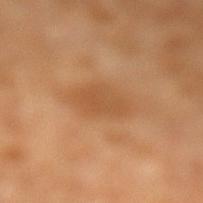Notes:
- follow-up: imaged on a skin check; not biopsied
- lesion diameter: ~4.5 mm (longest diameter)
- TBP lesion metrics: an area of roughly 10 mm², an outline eccentricity of about 0.75 (0 = round, 1 = elongated), and a symmetry-axis asymmetry near 0.15; a mean CIELAB color near L≈52 a*≈21 b*≈38, roughly 7 lightness units darker than nearby skin, and a lesion-to-skin contrast of about 5.5 (normalized; higher = more distinct); internal color variation of about 2.5 on a 0–10 scale and peripheral color asymmetry of about 1; lesion-presence confidence of about 100/100
- imaging modality: total-body-photography crop, ~15 mm field of view
- location: the left lower leg
- subject: male, approximately 30 years of age